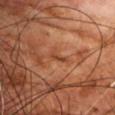This lesion was catalogued during total-body skin photography and was not selected for biopsy. The subject is a male aged 68 to 72. The lesion is on the chest. Captured under cross-polarized illumination. A 15 mm close-up tile from a total-body photography series done for melanoma screening.